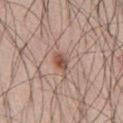Part of a total-body skin-imaging series; this lesion was reviewed on a skin check and was not flagged for biopsy.
The lesion is located on the abdomen.
Imaged with white-light lighting.
The patient is a male aged approximately 70.
A roughly 15 mm field-of-view crop from a total-body skin photograph.
About 2.5 mm across.